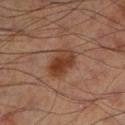The lesion was photographed on a routine skin check and not biopsied; there is no pathology result.
Approximately 4 mm at its widest.
The subject is a male about 70 years old.
Cropped from a total-body skin-imaging series; the visible field is about 15 mm.
The tile uses cross-polarized illumination.
On the leg.
The lesion-visualizer software estimated a lesion color around L≈31 a*≈19 b*≈25 in CIELAB, roughly 9 lightness units darker than nearby skin, and a lesion-to-skin contrast of about 9 (normalized; higher = more distinct). It also reported a classifier nevus-likeness of about 85/100.